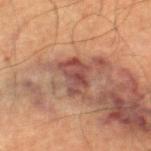notes = catalogued during a skin exam; not biopsied
image source = total-body-photography crop, ~15 mm field of view
illumination = cross-polarized
location = the right thigh
subject = male, aged 63 to 67